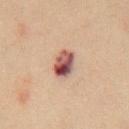biopsy_status: not biopsied; imaged during a skin examination
image:
  source: total-body photography crop
  field_of_view_mm: 15
patient:
  sex: female
  age_approx: 70
site: chest
lesion_size:
  long_diameter_mm_approx: 3.0